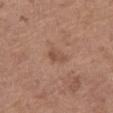follow-up: imaged on a skin check; not biopsied